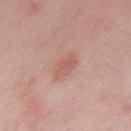biopsy status — imaged on a skin check; not biopsied | lesion size — ~4 mm (longest diameter) | lighting — white-light illumination | subject — male, aged 38 to 42 | location — the upper back | image source — ~15 mm tile from a whole-body skin photo.